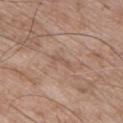  biopsy_status: not biopsied; imaged during a skin examination
  site: chest
  lesion_size:
    long_diameter_mm_approx: 3.0
  automated_metrics:
    area_mm2_approx: 2.0
    eccentricity: 0.95
    shape_asymmetry: 0.45
    cielab_L: 54
    cielab_a: 17
    cielab_b: 27
    vs_skin_contrast_norm: 4.5
  patient:
    sex: male
    age_approx: 50
  lighting: white-light
  image:
    source: total-body photography crop
    field_of_view_mm: 15